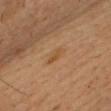Assessment: The lesion was tiled from a total-body skin photograph and was not biopsied. Context: Imaged with cross-polarized lighting. Located on the head or neck. A roughly 15 mm field-of-view crop from a total-body skin photograph. An algorithmic analysis of the crop reported an area of roughly 3 mm², an eccentricity of roughly 0.9, and a symmetry-axis asymmetry near 0.2. The analysis additionally found an average lesion color of about L≈44 a*≈18 b*≈34 (CIELAB) and a normalized border contrast of about 5.5. The software also gave a within-lesion color-variation index near 1/10. The software also gave a lesion-detection confidence of about 100/100. The patient is a male aged approximately 50. The lesion's longest dimension is about 2.5 mm.